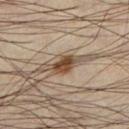On the leg. Measured at roughly 3 mm in maximum diameter. The lesion-visualizer software estimated radial color variation of about 1.5. And it measured a nevus-likeness score of about 95/100 and lesion-presence confidence of about 100/100. The subject is a male roughly 45 years of age. A 15 mm close-up tile from a total-body photography series done for melanoma screening. The tile uses cross-polarized illumination.